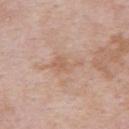The lesion was photographed on a routine skin check and not biopsied; there is no pathology result. A roughly 15 mm field-of-view crop from a total-body skin photograph. From the left upper arm. A male patient, aged 53–57.